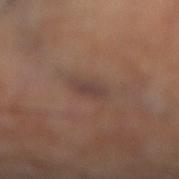Part of a total-body skin-imaging series; this lesion was reviewed on a skin check and was not flagged for biopsy. From the leg. Imaged with cross-polarized lighting. A 15 mm crop from a total-body photograph taken for skin-cancer surveillance. Automated tile analysis of the lesion measured a footprint of about 3.5 mm², an outline eccentricity of about 0.85 (0 = round, 1 = elongated), and two-axis asymmetry of about 0.2. The analysis additionally found a mean CIELAB color near L≈41 a*≈16 b*≈22, roughly 7 lightness units darker than nearby skin, and a normalized border contrast of about 6.5. About 3 mm across.